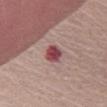Image and clinical context:
Located on the mid back. An algorithmic analysis of the crop reported a lesion color around L≈45 a*≈30 b*≈19 in CIELAB, a lesion–skin lightness drop of about 15, and a normalized lesion–skin contrast near 11. And it measured border irregularity of about 1.5 on a 0–10 scale and internal color variation of about 3 on a 0–10 scale. The patient is a female in their 80s. The lesion's longest dimension is about 2.5 mm. A 15 mm crop from a total-body photograph taken for skin-cancer surveillance. This is a white-light tile.